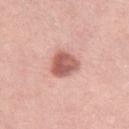Q: Illumination type?
A: white-light illumination
Q: What is the anatomic site?
A: the right thigh
Q: Patient demographics?
A: female, about 45 years old
Q: Lesion size?
A: ~4 mm (longest diameter)
Q: What is the imaging modality?
A: ~15 mm crop, total-body skin-cancer survey